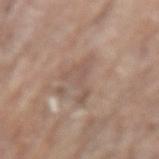follow-up — no biopsy performed (imaged during a skin exam).